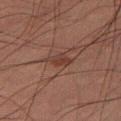A lesion tile, about 15 mm wide, cut from a 3D total-body photograph. Located on the left thigh. About 2.5 mm across. The tile uses cross-polarized illumination. The patient is a male about 55 years old.